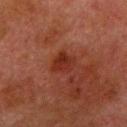Part of a total-body skin-imaging series; this lesion was reviewed on a skin check and was not flagged for biopsy. This is a cross-polarized tile. The total-body-photography lesion software estimated an outline eccentricity of about 0.35 (0 = round, 1 = elongated). And it measured border irregularity of about 2.5 on a 0–10 scale, internal color variation of about 3 on a 0–10 scale, and a peripheral color-asymmetry measure near 1. Located on the right lower leg. Approximately 3 mm at its widest. A male patient aged 78–82. A roughly 15 mm field-of-view crop from a total-body skin photograph.